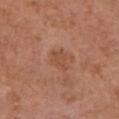Q: Is there a histopathology result?
A: imaged on a skin check; not biopsied
Q: What is the anatomic site?
A: the front of the torso
Q: Who is the patient?
A: female, roughly 80 years of age
Q: What kind of image is this?
A: total-body-photography crop, ~15 mm field of view
Q: What did automated image analysis measure?
A: a lesion color around L≈49 a*≈23 b*≈32 in CIELAB, about 7 CIELAB-L* units darker than the surrounding skin, and a lesion-to-skin contrast of about 5 (normalized; higher = more distinct); an automated nevus-likeness rating near 0 out of 100 and a detector confidence of about 100 out of 100 that the crop contains a lesion